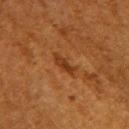Clinical summary: A female subject, aged 48 to 52. From the right upper arm. Measured at roughly 3.5 mm in maximum diameter. This is a cross-polarized tile. A 15 mm close-up tile from a total-body photography series done for melanoma screening.